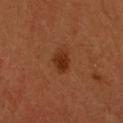Assessment:
The lesion was photographed on a routine skin check and not biopsied; there is no pathology result.
Background:
About 3 mm across. The subject is a female aged 48 to 52. Imaged with cross-polarized lighting. A close-up tile cropped from a whole-body skin photograph, about 15 mm across.